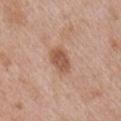Recorded during total-body skin imaging; not selected for excision or biopsy.
From the right upper arm.
A region of skin cropped from a whole-body photographic capture, roughly 15 mm wide.
The lesion's longest dimension is about 3.5 mm.
The subject is a male aged 48–52.
An algorithmic analysis of the crop reported a lesion color around L≈55 a*≈21 b*≈30 in CIELAB, a lesion–skin lightness drop of about 11, and a lesion-to-skin contrast of about 8 (normalized; higher = more distinct). The analysis additionally found a border-irregularity index near 1.5/10, a within-lesion color-variation index near 2.5/10, and a peripheral color-asymmetry measure near 1.